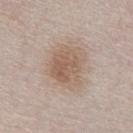Impression: Imaged during a routine full-body skin examination; the lesion was not biopsied and no histopathology is available. Context: Captured under white-light illumination. On the chest. A male subject aged 63 to 67. About 5 mm across. Cropped from a whole-body photographic skin survey; the tile spans about 15 mm.